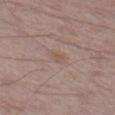Q: Was a biopsy performed?
A: imaged on a skin check; not biopsied
Q: What is the imaging modality?
A: 15 mm crop, total-body photography
Q: Patient demographics?
A: male, aged 73 to 77
Q: Where on the body is the lesion?
A: the left thigh